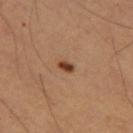Assessment:
No biopsy was performed on this lesion — it was imaged during a full skin examination and was not determined to be concerning.
Clinical summary:
The subject is a female aged approximately 55. The lesion is on the leg. A lesion tile, about 15 mm wide, cut from a 3D total-body photograph. The lesion-visualizer software estimated an area of roughly 2 mm² and an outline eccentricity of about 0.8 (0 = round, 1 = elongated). The software also gave an average lesion color of about L≈41 a*≈22 b*≈33 (CIELAB), about 15 CIELAB-L* units darker than the surrounding skin, and a normalized lesion–skin contrast near 11.5. This is a cross-polarized tile. Longest diameter approximately 2 mm.